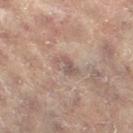workup: imaged on a skin check; not biopsied
subject: female, aged 78–82
anatomic site: the left leg
acquisition: ~15 mm crop, total-body skin-cancer survey